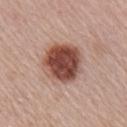Captured during whole-body skin photography for melanoma surveillance; the lesion was not biopsied. An algorithmic analysis of the crop reported a lesion area of about 19 mm², an outline eccentricity of about 0.35 (0 = round, 1 = elongated), and two-axis asymmetry of about 0.1. And it measured a mean CIELAB color near L≈48 a*≈23 b*≈26 and a normalized border contrast of about 12.5. And it measured a border-irregularity index near 1/10, a within-lesion color-variation index near 6.5/10, and a peripheral color-asymmetry measure near 2. The analysis additionally found a nevus-likeness score of about 95/100. Captured under white-light illumination. The patient is a male in their mid- to late 60s. Measured at roughly 5 mm in maximum diameter. The lesion is on the right upper arm. Cropped from a whole-body photographic skin survey; the tile spans about 15 mm.